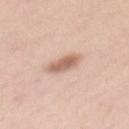Case summary:
• biopsy status · imaged on a skin check; not biopsied
• site · the mid back
• image source · ~15 mm crop, total-body skin-cancer survey
• lesion size · ~3.5 mm (longest diameter)
• automated lesion analysis · border irregularity of about 2 on a 0–10 scale, internal color variation of about 2.5 on a 0–10 scale, and peripheral color asymmetry of about 1; a classifier nevus-likeness of about 75/100 and a detector confidence of about 100 out of 100 that the crop contains a lesion
• subject · female, roughly 35 years of age